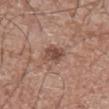Imaged during a routine full-body skin examination; the lesion was not biopsied and no histopathology is available.
A 15 mm crop from a total-body photograph taken for skin-cancer surveillance.
A male patient roughly 75 years of age.
Longest diameter approximately 2.5 mm.
An algorithmic analysis of the crop reported a lesion area of about 5.5 mm², an outline eccentricity of about 0.45 (0 = round, 1 = elongated), and two-axis asymmetry of about 0.2. The software also gave a lesion color around L≈47 a*≈20 b*≈25 in CIELAB, a lesion–skin lightness drop of about 11, and a lesion-to-skin contrast of about 8 (normalized; higher = more distinct). The software also gave a detector confidence of about 100 out of 100 that the crop contains a lesion.
Located on the front of the torso.